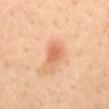Impression: No biopsy was performed on this lesion — it was imaged during a full skin examination and was not determined to be concerning. Context: Measured at roughly 2.5 mm in maximum diameter. A 15 mm close-up tile from a total-body photography series done for melanoma screening. Automated tile analysis of the lesion measured a mean CIELAB color near L≈65 a*≈27 b*≈38, about 10 CIELAB-L* units darker than the surrounding skin, and a normalized border contrast of about 6.5. The analysis additionally found a classifier nevus-likeness of about 60/100 and a lesion-detection confidence of about 100/100. A male patient, aged 38–42. This is a cross-polarized tile. From the mid back.